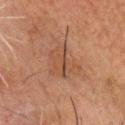<lesion>
<site>head or neck</site>
<patient>
  <sex>male</sex>
  <age_approx>80</age_approx>
</patient>
<image>
  <source>total-body photography crop</source>
  <field_of_view_mm>15</field_of_view_mm>
</image>
</lesion>